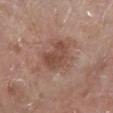biopsy status: total-body-photography surveillance lesion; no biopsy
diameter: ~5 mm (longest diameter)
acquisition: ~15 mm crop, total-body skin-cancer survey
subject: male, aged 78–82
location: the right lower leg